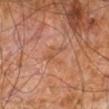Imaged during a routine full-body skin examination; the lesion was not biopsied and no histopathology is available.
About 3 mm across.
A male patient about 60 years old.
From the right leg.
Cropped from a total-body skin-imaging series; the visible field is about 15 mm.
Imaged with cross-polarized lighting.
Automated tile analysis of the lesion measured a footprint of about 4.5 mm² and a shape eccentricity near 0.65. And it measured a border-irregularity rating of about 6.5/10, a within-lesion color-variation index near 2/10, and radial color variation of about 0.5.